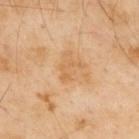Clinical impression: No biopsy was performed on this lesion — it was imaged during a full skin examination and was not determined to be concerning. Acquisition and patient details: From the upper back. The tile uses cross-polarized illumination. A 15 mm close-up tile from a total-body photography series done for melanoma screening. A male subject, aged 53 to 57.